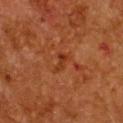Impression: Recorded during total-body skin imaging; not selected for excision or biopsy. Clinical summary: A female subject, aged around 50. A close-up tile cropped from a whole-body skin photograph, about 15 mm across. The lesion is on the front of the torso.